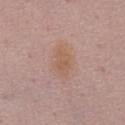The lesion was tiled from a total-body skin photograph and was not biopsied. A roughly 15 mm field-of-view crop from a total-body skin photograph. This is a white-light tile. Automated tile analysis of the lesion measured a footprint of about 7 mm², an eccentricity of roughly 0.8, and two-axis asymmetry of about 0.25. It also reported a lesion color around L≈57 a*≈19 b*≈29 in CIELAB, roughly 6 lightness units darker than nearby skin, and a normalized border contrast of about 6.5. The analysis additionally found a classifier nevus-likeness of about 15/100 and a detector confidence of about 100 out of 100 that the crop contains a lesion. Measured at roughly 4 mm in maximum diameter. The lesion is located on the chest. The patient is a female in their 50s.